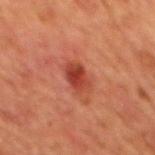Impression:
No biopsy was performed on this lesion — it was imaged during a full skin examination and was not determined to be concerning.
Acquisition and patient details:
About 3.5 mm across. Imaged with cross-polarized lighting. A male patient, aged 63 to 67. From the back. A lesion tile, about 15 mm wide, cut from a 3D total-body photograph.